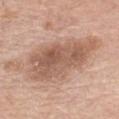No biopsy was performed on this lesion — it was imaged during a full skin examination and was not determined to be concerning. A female patient, aged around 75. Located on the chest. A roughly 15 mm field-of-view crop from a total-body skin photograph. This is a white-light tile. Longest diameter approximately 9.5 mm.